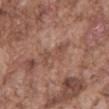Q: Is there a histopathology result?
A: total-body-photography surveillance lesion; no biopsy
Q: What is the anatomic site?
A: the mid back
Q: What kind of image is this?
A: ~15 mm tile from a whole-body skin photo
Q: Automated lesion metrics?
A: a mean CIELAB color near L≈49 a*≈21 b*≈27, roughly 7 lightness units darker than nearby skin, and a normalized border contrast of about 5
Q: Who is the patient?
A: male, in their mid-70s